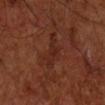Assessment:
No biopsy was performed on this lesion — it was imaged during a full skin examination and was not determined to be concerning.
Context:
A male subject, aged approximately 60. Captured under cross-polarized illumination. A lesion tile, about 15 mm wide, cut from a 3D total-body photograph. Approximately 5 mm at its widest. The lesion is located on the right forearm.